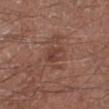The lesion was photographed on a routine skin check and not biopsied; there is no pathology result.
The lesion is on the left lower leg.
This image is a 15 mm lesion crop taken from a total-body photograph.
Approximately 3 mm at its widest.
Imaged with white-light lighting.
A male subject in their mid-50s.